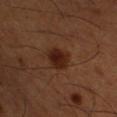Captured during whole-body skin photography for melanoma surveillance; the lesion was not biopsied.
A close-up tile cropped from a whole-body skin photograph, about 15 mm across.
Imaged with cross-polarized lighting.
From the right lower leg.
Approximately 2.5 mm at its widest.
A male patient aged 48 to 52.